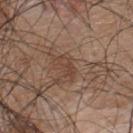biopsy_status: not biopsied; imaged during a skin examination
image:
  source: total-body photography crop
  field_of_view_mm: 15
site: upper back
patient:
  sex: male
  age_approx: 45